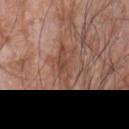notes = imaged on a skin check; not biopsied | image = 15 mm crop, total-body photography | diameter = ~5 mm (longest diameter) | subject = male, aged 58–62 | lighting = white-light | site = the left forearm.